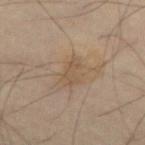This lesion was catalogued during total-body skin photography and was not selected for biopsy. This is a cross-polarized tile. The lesion's longest dimension is about 3 mm. Cropped from a total-body skin-imaging series; the visible field is about 15 mm. The total-body-photography lesion software estimated an eccentricity of roughly 0.9 and two-axis asymmetry of about 0.4. The analysis additionally found a lesion color around L≈49 a*≈13 b*≈29 in CIELAB, roughly 6 lightness units darker than nearby skin, and a normalized lesion–skin contrast near 5. It also reported a nevus-likeness score of about 0/100 and a lesion-detection confidence of about 100/100. The lesion is located on the front of the torso. A male subject aged 63–67.